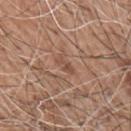The lesion was photographed on a routine skin check and not biopsied; there is no pathology result.
The subject is a male aged 58 to 62.
Captured under white-light illumination.
On the right upper arm.
A roughly 15 mm field-of-view crop from a total-body skin photograph.
Approximately 2.5 mm at its widest.